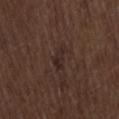{"patient": {"sex": "male", "age_approx": 70}, "site": "back", "image": {"source": "total-body photography crop", "field_of_view_mm": 15}, "lighting": "white-light"}